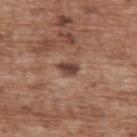notes — total-body-photography surveillance lesion; no biopsy
diameter — ≈2.5 mm
location — the upper back
acquisition — 15 mm crop, total-body photography
patient — male, aged 68–72